The recorded lesion diameter is about 5.5 mm. Captured under white-light illumination. A lesion tile, about 15 mm wide, cut from a 3D total-body photograph. On the chest. A female patient about 65 years old. The lesion-visualizer software estimated an area of roughly 16 mm², a shape eccentricity near 0.7, and a symmetry-axis asymmetry near 0.25. It also reported internal color variation of about 6 on a 0–10 scale and peripheral color asymmetry of about 2. The software also gave a classifier nevus-likeness of about 70/100 and lesion-presence confidence of about 100/100 — 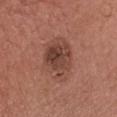diagnosis = a seborrheic keratosis (benign).